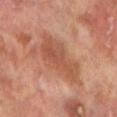Clinical impression:
Recorded during total-body skin imaging; not selected for excision or biopsy.
Clinical summary:
The subject is a male roughly 70 years of age. A 15 mm close-up tile from a total-body photography series done for melanoma screening. On the left lower leg. Captured under cross-polarized illumination. The lesion's longest dimension is about 6.5 mm.